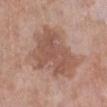Impression:
The lesion was tiled from a total-body skin photograph and was not biopsied.
Acquisition and patient details:
Approximately 7.5 mm at its widest. The lesion is on the left lower leg. Automated tile analysis of the lesion measured a lesion area of about 29 mm², an outline eccentricity of about 0.7 (0 = round, 1 = elongated), and two-axis asymmetry of about 0.35. The software also gave a mean CIELAB color near L≈53 a*≈20 b*≈27, about 10 CIELAB-L* units darker than the surrounding skin, and a lesion-to-skin contrast of about 7 (normalized; higher = more distinct). The software also gave a nevus-likeness score of about 0/100 and lesion-presence confidence of about 100/100. A female patient, in their mid-50s. Cropped from a total-body skin-imaging series; the visible field is about 15 mm. Imaged with white-light lighting.